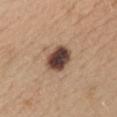- biopsy status · no biopsy performed (imaged during a skin exam)
- image · 15 mm crop, total-body photography
- patient · female, aged approximately 45
- lighting · white-light
- body site · the chest